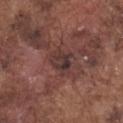Assessment: Recorded during total-body skin imaging; not selected for excision or biopsy. Image and clinical context: A male patient, aged 73–77. This image is a 15 mm lesion crop taken from a total-body photograph. On the chest. Measured at roughly 3.5 mm in maximum diameter.